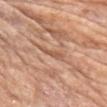Acquisition and patient details:
The recorded lesion diameter is about 2.5 mm. From the left upper arm. This is a white-light tile. A female subject approximately 75 years of age. The lesion-visualizer software estimated a footprint of about 3 mm², a shape eccentricity near 0.9, and a symmetry-axis asymmetry near 0.4. The analysis additionally found a lesion color around L≈56 a*≈22 b*≈32 in CIELAB, about 9 CIELAB-L* units darker than the surrounding skin, and a lesion-to-skin contrast of about 6 (normalized; higher = more distinct). It also reported a border-irregularity index near 4/10 and a color-variation rating of about 1/10. A region of skin cropped from a whole-body photographic capture, roughly 15 mm wide.